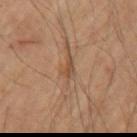Part of a total-body skin-imaging series; this lesion was reviewed on a skin check and was not flagged for biopsy. A male subject, about 60 years old. A 15 mm close-up tile from a total-body photography series done for melanoma screening. Captured under cross-polarized illumination. The recorded lesion diameter is about 3 mm. The lesion is on the arm.